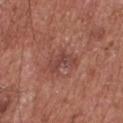  automated_metrics:
    border_irregularity_0_10: 4.5
    color_variation_0_10: 3.0
  patient:
    sex: male
    age_approx: 75
  image:
    source: total-body photography crop
    field_of_view_mm: 15
  lesion_size:
    long_diameter_mm_approx: 4.0
  site: chest